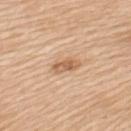Captured under white-light illumination. The recorded lesion diameter is about 3.5 mm. A female subject, aged 53–57. Cropped from a total-body skin-imaging series; the visible field is about 15 mm. On the upper back.